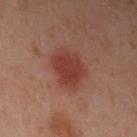image — 15 mm crop, total-body photography | lesion size — ≈5 mm | body site — the left upper arm | subject — female, aged around 40 | automated metrics — a lesion area of about 14 mm², an eccentricity of roughly 0.55, and two-axis asymmetry of about 0.15; an average lesion color of about L≈41 a*≈27 b*≈28 (CIELAB), roughly 9 lightness units darker than nearby skin, and a normalized border contrast of about 8; a nevus-likeness score of about 100/100 and lesion-presence confidence of about 100/100.